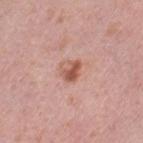Clinical impression:
The lesion was tiled from a total-body skin photograph and was not biopsied.
Acquisition and patient details:
Imaged with white-light lighting. Located on the left thigh. A female subject approximately 40 years of age. A lesion tile, about 15 mm wide, cut from a 3D total-body photograph. Approximately 2.5 mm at its widest.